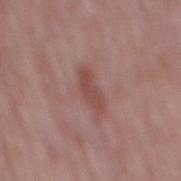The lesion was tiled from a total-body skin photograph and was not biopsied. Cropped from a whole-body photographic skin survey; the tile spans about 15 mm. Captured under white-light illumination. The patient is a male aged 63–67. From the back. The recorded lesion diameter is about 4 mm.